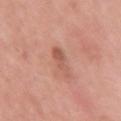Clinical impression:
Imaged during a routine full-body skin examination; the lesion was not biopsied and no histopathology is available.
Background:
The subject is a female aged around 70. An algorithmic analysis of the crop reported an automated nevus-likeness rating near 5 out of 100 and lesion-presence confidence of about 100/100. On the right upper arm. The lesion's longest dimension is about 3 mm. A 15 mm close-up extracted from a 3D total-body photography capture.